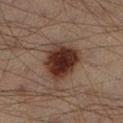Findings:
– notes · imaged on a skin check; not biopsied
– diameter · ~5 mm (longest diameter)
– imaging modality · total-body-photography crop, ~15 mm field of view
– illumination · cross-polarized
– subject · male, aged 38 to 42
– anatomic site · the left lower leg